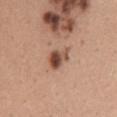This lesion was catalogued during total-body skin photography and was not selected for biopsy.
The lesion is located on the mid back.
A female patient, aged approximately 35.
A 15 mm close-up extracted from a 3D total-body photography capture.
The recorded lesion diameter is about 3.5 mm.